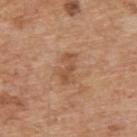Notes:
• follow-up · imaged on a skin check; not biopsied
• imaging modality · ~15 mm crop, total-body skin-cancer survey
• TBP lesion metrics · a border-irregularity rating of about 5/10 and a peripheral color-asymmetry measure near 0.5; a nevus-likeness score of about 0/100 and lesion-presence confidence of about 100/100
• subject · male, aged approximately 65
• anatomic site · the upper back
• lighting · white-light illumination
• lesion diameter · ≈3.5 mm